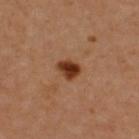  biopsy_status: not biopsied; imaged during a skin examination
  patient:
    sex: female
    age_approx: 45
  image:
    source: total-body photography crop
    field_of_view_mm: 15
  lesion_size:
    long_diameter_mm_approx: 3.0
  lighting: cross-polarized
  site: upper back